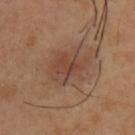Captured during whole-body skin photography for melanoma surveillance; the lesion was not biopsied. Imaged with cross-polarized lighting. A male patient, aged 38 to 42. Located on the upper back. Cropped from a whole-body photographic skin survey; the tile spans about 15 mm. The lesion-visualizer software estimated a footprint of about 13 mm². The analysis additionally found a classifier nevus-likeness of about 70/100 and a lesion-detection confidence of about 100/100.